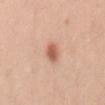Assessment: This lesion was catalogued during total-body skin photography and was not selected for biopsy. Acquisition and patient details: A 15 mm crop from a total-body photograph taken for skin-cancer surveillance. The lesion is located on the lower back. Captured under white-light illumination. A male subject, in their mid-20s. Approximately 3 mm at its widest.